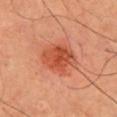| key | value |
|---|---|
| follow-up | imaged on a skin check; not biopsied |
| patient | male, approximately 65 years of age |
| image source | total-body-photography crop, ~15 mm field of view |
| lighting | cross-polarized illumination |
| image-analysis metrics | a footprint of about 13 mm², a shape eccentricity near 0.65, and a shape-asymmetry score of about 0.2 (0 = symmetric); internal color variation of about 5 on a 0–10 scale and a peripheral color-asymmetry measure near 1.5 |
| location | the front of the torso |